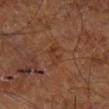Clinical impression:
Recorded during total-body skin imaging; not selected for excision or biopsy.
Acquisition and patient details:
The tile uses cross-polarized illumination. The lesion's longest dimension is about 3.5 mm. A subject in their mid-60s. On the right lower leg. This image is a 15 mm lesion crop taken from a total-body photograph.